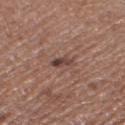Captured during whole-body skin photography for melanoma surveillance; the lesion was not biopsied.
An algorithmic analysis of the crop reported an outline eccentricity of about 0.85 (0 = round, 1 = elongated) and two-axis asymmetry of about 0.3. The analysis additionally found a mean CIELAB color near L≈42 a*≈19 b*≈21, a lesion–skin lightness drop of about 11, and a normalized border contrast of about 9.
Cropped from a total-body skin-imaging series; the visible field is about 15 mm.
On the left lower leg.
Imaged with white-light lighting.
A female subject, aged approximately 50.